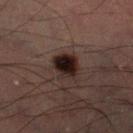Impression: The lesion was tiled from a total-body skin photograph and was not biopsied. Background: A male patient, in their 60s. Cropped from a total-body skin-imaging series; the visible field is about 15 mm. An algorithmic analysis of the crop reported a lesion area of about 7.5 mm² and two-axis asymmetry of about 0.2. The software also gave a lesion color around L≈17 a*≈12 b*≈14 in CIELAB, roughly 13 lightness units darker than nearby skin, and a lesion-to-skin contrast of about 15.5 (normalized; higher = more distinct). And it measured border irregularity of about 2 on a 0–10 scale, a color-variation rating of about 6/10, and a peripheral color-asymmetry measure near 1.5.